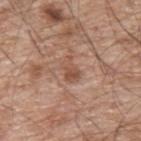Clinical impression:
This lesion was catalogued during total-body skin photography and was not selected for biopsy.
Acquisition and patient details:
Cropped from a whole-body photographic skin survey; the tile spans about 15 mm. The lesion-visualizer software estimated about 8 CIELAB-L* units darker than the surrounding skin and a normalized border contrast of about 6. The software also gave border irregularity of about 4 on a 0–10 scale, a within-lesion color-variation index near 3.5/10, and peripheral color asymmetry of about 1.5. The analysis additionally found a classifier nevus-likeness of about 0/100 and a lesion-detection confidence of about 100/100. The recorded lesion diameter is about 3 mm. The lesion is on the upper back. A male subject about 65 years old. Captured under white-light illumination.